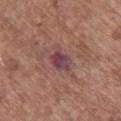Case summary:
* automated lesion analysis — an area of roughly 6 mm² and two-axis asymmetry of about 0.3; a mean CIELAB color near L≈43 a*≈24 b*≈16 and a lesion-to-skin contrast of about 10 (normalized; higher = more distinct); a border-irregularity index near 3/10, a within-lesion color-variation index near 5.5/10, and a peripheral color-asymmetry measure near 2
* size — ~3 mm (longest diameter)
* illumination — white-light illumination
* patient — female, in their mid- to late 70s
* site — the upper back
* image — ~15 mm crop, total-body skin-cancer survey